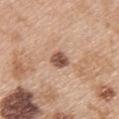Q: Was this lesion biopsied?
A: catalogued during a skin exam; not biopsied
Q: What lighting was used for the tile?
A: white-light
Q: What is the anatomic site?
A: the upper back
Q: Automated lesion metrics?
A: a footprint of about 4 mm², a shape eccentricity near 0.55, and a shape-asymmetry score of about 0.15 (0 = symmetric); about 16 CIELAB-L* units darker than the surrounding skin and a normalized border contrast of about 10.5
Q: Patient demographics?
A: female, approximately 45 years of age
Q: What is the lesion's diameter?
A: about 2.5 mm
Q: What is the imaging modality?
A: total-body-photography crop, ~15 mm field of view